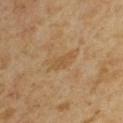Imaged during a routine full-body skin examination; the lesion was not biopsied and no histopathology is available.
The lesion's longest dimension is about 3 mm.
A male subject, in their mid- to late 60s.
A lesion tile, about 15 mm wide, cut from a 3D total-body photograph.
The total-body-photography lesion software estimated a lesion color around L≈51 a*≈17 b*≈36 in CIELAB, about 6 CIELAB-L* units darker than the surrounding skin, and a lesion-to-skin contrast of about 5 (normalized; higher = more distinct).
Captured under cross-polarized illumination.